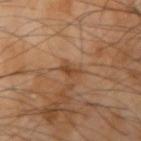Impression: This lesion was catalogued during total-body skin photography and was not selected for biopsy. Clinical summary: Measured at roughly 2.5 mm in maximum diameter. A male patient, aged 63 to 67. This is a cross-polarized tile. A 15 mm crop from a total-body photograph taken for skin-cancer surveillance. Located on the arm.